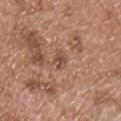Notes:
– location: the chest
– patient: male, approximately 55 years of age
– TBP lesion metrics: a lesion color around L≈50 a*≈21 b*≈27 in CIELAB and a lesion–skin lightness drop of about 8; a border-irregularity index near 2.5/10 and a color-variation rating of about 3/10
– acquisition: total-body-photography crop, ~15 mm field of view
– tile lighting: white-light illumination
– lesion size: ~2.5 mm (longest diameter)